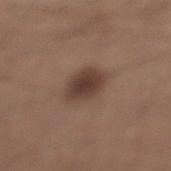{
  "biopsy_status": "not biopsied; imaged during a skin examination",
  "automated_metrics": {
    "cielab_L": 39,
    "cielab_a": 17,
    "cielab_b": 23,
    "color_variation_0_10": 3.5,
    "peripheral_color_asymmetry": 1.0
  },
  "image": {
    "source": "total-body photography crop",
    "field_of_view_mm": 15
  },
  "lesion_size": {
    "long_diameter_mm_approx": 4.0
  },
  "site": "leg",
  "patient": {
    "sex": "male",
    "age_approx": 40
  },
  "lighting": "white-light"
}